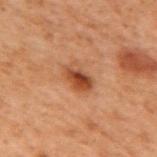{"image": {"source": "total-body photography crop", "field_of_view_mm": 15}, "lesion_size": {"long_diameter_mm_approx": 3.5}, "site": "mid back", "automated_metrics": {"area_mm2_approx": 7.0, "eccentricity": 0.75, "shape_asymmetry": 0.2, "border_irregularity_0_10": 2.0, "peripheral_color_asymmetry": 2.0, "nevus_likeness_0_100": 90, "lesion_detection_confidence_0_100": 100}, "patient": {"sex": "male", "age_approx": 70}}